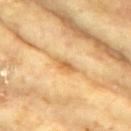Approximately 3 mm at its widest. The lesion is on the arm. Imaged with cross-polarized lighting. Cropped from a total-body skin-imaging series; the visible field is about 15 mm. The subject is a female aged 73 to 77.